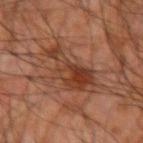A region of skin cropped from a whole-body photographic capture, roughly 15 mm wide. From the right forearm. A male subject aged around 65.